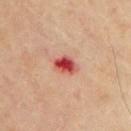Notes:
• tile lighting — cross-polarized
• patient — male, aged 58 to 62
• image-analysis metrics — a lesion area of about 5.5 mm², an outline eccentricity of about 0.7 (0 = round, 1 = elongated), and two-axis asymmetry of about 0.15; a lesion color around L≈46 a*≈33 b*≈27 in CIELAB, roughly 15 lightness units darker than nearby skin, and a normalized lesion–skin contrast near 10.5; a classifier nevus-likeness of about 0/100 and a lesion-detection confidence of about 100/100
• image — ~15 mm tile from a whole-body skin photo
• location — the chest